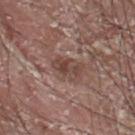Q: Is there a histopathology result?
A: no biopsy performed (imaged during a skin exam)
Q: How was this image acquired?
A: ~15 mm tile from a whole-body skin photo
Q: Lesion location?
A: the upper back
Q: How large is the lesion?
A: about 4 mm
Q: Who is the patient?
A: male, approximately 40 years of age
Q: How was the tile lit?
A: white-light